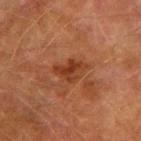Background:
The lesion's longest dimension is about 3.5 mm. A roughly 15 mm field-of-view crop from a total-body skin photograph. A male subject, aged 73–77. The tile uses cross-polarized illumination. On the arm. An algorithmic analysis of the crop reported an area of roughly 6.5 mm², an eccentricity of roughly 0.7, and two-axis asymmetry of about 0.4. It also reported a lesion color around L≈32 a*≈23 b*≈30 in CIELAB, about 8 CIELAB-L* units darker than the surrounding skin, and a lesion-to-skin contrast of about 7.5 (normalized; higher = more distinct).